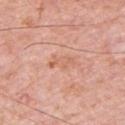body site = the left upper arm
subject = male, aged 78 to 82
image source = ~15 mm tile from a whole-body skin photo
size = ≈3.5 mm